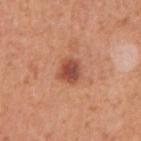follow-up=imaged on a skin check; not biopsied
acquisition=total-body-photography crop, ~15 mm field of view
lesion size=about 3 mm
subject=male, aged approximately 55
TBP lesion metrics=a lesion area of about 6 mm², a shape eccentricity near 0.6, and two-axis asymmetry of about 0.15; a border-irregularity index near 1.5/10, a within-lesion color-variation index near 4.5/10, and peripheral color asymmetry of about 1.5; a classifier nevus-likeness of about 90/100 and a lesion-detection confidence of about 100/100
site=the right upper arm